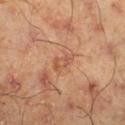<case>
  <lighting>cross-polarized</lighting>
  <site>right lower leg</site>
  <lesion_size>
    <long_diameter_mm_approx>3.0</long_diameter_mm_approx>
  </lesion_size>
  <patient>
    <sex>male</sex>
    <age_approx>45</age_approx>
  </patient>
  <image>
    <source>total-body photography crop</source>
    <field_of_view_mm>15</field_of_view_mm>
  </image>
</case>